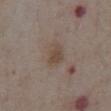| field | value |
|---|---|
| biopsy status | total-body-photography surveillance lesion; no biopsy |
| anatomic site | the abdomen |
| TBP lesion metrics | an area of roughly 5 mm²; a mean CIELAB color near L≈45 a*≈13 b*≈24 and a normalized border contrast of about 6.5 |
| patient | male, approximately 75 years of age |
| diameter | ≈3 mm |
| image source | ~15 mm crop, total-body skin-cancer survey |